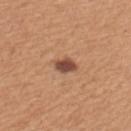Imaged during a routine full-body skin examination; the lesion was not biopsied and no histopathology is available.
Captured under white-light illumination.
On the upper back.
About 3 mm across.
A 15 mm crop from a total-body photograph taken for skin-cancer surveillance.
A female subject in their 30s.
The total-body-photography lesion software estimated a shape-asymmetry score of about 0.25 (0 = symmetric). And it measured lesion-presence confidence of about 100/100.